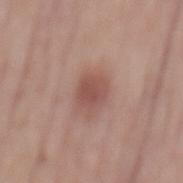This lesion was catalogued during total-body skin photography and was not selected for biopsy. The lesion is located on the back. A male patient, aged approximately 60. A region of skin cropped from a whole-body photographic capture, roughly 15 mm wide. The lesion's longest dimension is about 3 mm. Captured under white-light illumination.